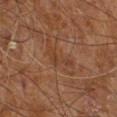Case summary:
– workup · catalogued during a skin exam; not biopsied
– lesion size · about 4 mm
– body site · the right leg
– acquisition · ~15 mm tile from a whole-body skin photo
– subject · male, about 60 years old
– lighting · cross-polarized illumination